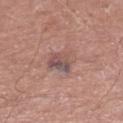Part of a total-body skin-imaging series; this lesion was reviewed on a skin check and was not flagged for biopsy. A male subject aged 68–72. From the right lower leg. Automated tile analysis of the lesion measured a lesion area of about 9.5 mm² and an outline eccentricity of about 0.4 (0 = round, 1 = elongated). It also reported a mean CIELAB color near L≈52 a*≈19 b*≈21 and about 8 CIELAB-L* units darker than the surrounding skin. And it measured a nevus-likeness score of about 0/100 and a lesion-detection confidence of about 95/100. A 15 mm close-up extracted from a 3D total-body photography capture.